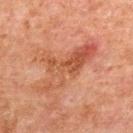Part of a total-body skin-imaging series; this lesion was reviewed on a skin check and was not flagged for biopsy.